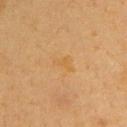– workup — imaged on a skin check; not biopsied
– subject — female, aged 38 to 42
– site — the upper back
– image — ~15 mm tile from a whole-body skin photo